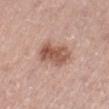Impression:
No biopsy was performed on this lesion — it was imaged during a full skin examination and was not determined to be concerning.
Context:
Longest diameter approximately 4.5 mm. Automated image analysis of the tile measured a border-irregularity rating of about 2/10 and a within-lesion color-variation index near 5.5/10. The software also gave a classifier nevus-likeness of about 45/100. The lesion is located on the right thigh. Cropped from a whole-body photographic skin survey; the tile spans about 15 mm. The tile uses white-light illumination. The subject is a male in their mid-70s.